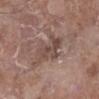<lesion>
<biopsy_status>not biopsied; imaged during a skin examination</biopsy_status>
<lighting>white-light</lighting>
<patient>
  <sex>female</sex>
  <age_approx>75</age_approx>
</patient>
<lesion_size>
  <long_diameter_mm_approx>5.5</long_diameter_mm_approx>
</lesion_size>
<image>
  <source>total-body photography crop</source>
  <field_of_view_mm>15</field_of_view_mm>
</image>
<site>right lower leg</site>
</lesion>